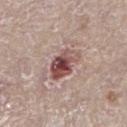The lesion was photographed on a routine skin check and not biopsied; there is no pathology result.
Imaged with white-light lighting.
A male subject, in their mid-70s.
A lesion tile, about 15 mm wide, cut from a 3D total-body photograph.
The lesion is located on the left lower leg.
Longest diameter approximately 4 mm.
Automated tile analysis of the lesion measured a lesion area of about 9 mm², an outline eccentricity of about 0.75 (0 = round, 1 = elongated), and a shape-asymmetry score of about 0.3 (0 = symmetric).